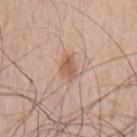A region of skin cropped from a whole-body photographic capture, roughly 15 mm wide. From the chest. A male patient aged 63 to 67. Longest diameter approximately 3 mm.